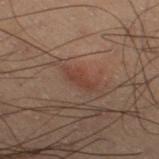| key | value |
|---|---|
| workup | no biopsy performed (imaged during a skin exam) |
| tile lighting | cross-polarized illumination |
| automated lesion analysis | a lesion area of about 4 mm², a shape eccentricity near 0.95, and a symmetry-axis asymmetry near 0.3; roughly 5 lightness units darker than nearby skin and a normalized lesion–skin contrast near 5.5 |
| site | the leg |
| lesion diameter | ~3.5 mm (longest diameter) |
| imaging modality | 15 mm crop, total-body photography |
| patient | male, aged 53–57 |